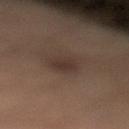The lesion was photographed on a routine skin check and not biopsied; there is no pathology result.
The lesion is located on the right leg.
A region of skin cropped from a whole-body photographic capture, roughly 15 mm wide.
A male patient, aged approximately 35.
Imaged with cross-polarized lighting.